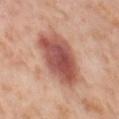notes=no biopsy performed (imaged during a skin exam).